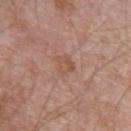The lesion was tiled from a total-body skin photograph and was not biopsied. The lesion is located on the left upper arm. A 15 mm close-up extracted from a 3D total-body photography capture. Captured under white-light illumination. The lesion's longest dimension is about 2.5 mm. The patient is a male in their mid- to late 60s.